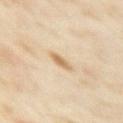Assessment:
Part of a total-body skin-imaging series; this lesion was reviewed on a skin check and was not flagged for biopsy.
Context:
Located on the right upper arm. Captured under cross-polarized illumination. A female patient, in their mid-30s. A lesion tile, about 15 mm wide, cut from a 3D total-body photograph. Automated image analysis of the tile measured a lesion color around L≈65 a*≈15 b*≈36 in CIELAB, about 10 CIELAB-L* units darker than the surrounding skin, and a lesion-to-skin contrast of about 7 (normalized; higher = more distinct). About 2.5 mm across.